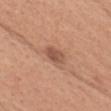  biopsy_status: not biopsied; imaged during a skin examination
  lighting: white-light
  automated_metrics:
    vs_skin_darker_L: 11.0
    border_irregularity_0_10: 2.0
    color_variation_0_10: 3.0
    peripheral_color_asymmetry: 1.0
    nevus_likeness_0_100: 20
    lesion_detection_confidence_0_100: 100
  image:
    source: total-body photography crop
    field_of_view_mm: 15
  patient:
    sex: female
    age_approx: 40
  lesion_size:
    long_diameter_mm_approx: 3.0
  site: head or neck